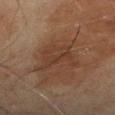Q: Is there a histopathology result?
A: catalogued during a skin exam; not biopsied
Q: Where on the body is the lesion?
A: the left lower leg
Q: Patient demographics?
A: male, aged 68–72
Q: How was the tile lit?
A: cross-polarized
Q: What kind of image is this?
A: ~15 mm tile from a whole-body skin photo
Q: How large is the lesion?
A: about 5 mm